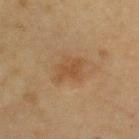Clinical impression:
Part of a total-body skin-imaging series; this lesion was reviewed on a skin check and was not flagged for biopsy.
Context:
The total-body-photography lesion software estimated a footprint of about 7.5 mm² and two-axis asymmetry of about 0.3. It also reported about 6 CIELAB-L* units darker than the surrounding skin and a lesion-to-skin contrast of about 5.5 (normalized; higher = more distinct). The software also gave a border-irregularity rating of about 3/10 and a within-lesion color-variation index near 2/10. The analysis additionally found lesion-presence confidence of about 100/100. Longest diameter approximately 3.5 mm. From the right upper arm. A close-up tile cropped from a whole-body skin photograph, about 15 mm across. The patient is a female aged 68 to 72.